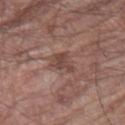Captured during whole-body skin photography for melanoma surveillance; the lesion was not biopsied. A male patient, in their 80s. A lesion tile, about 15 mm wide, cut from a 3D total-body photograph. The lesion is on the right thigh. The recorded lesion diameter is about 3 mm. Captured under white-light illumination.